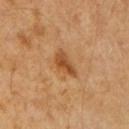| feature | finding |
|---|---|
| biopsy status | imaged on a skin check; not biopsied |
| subject | male, in their 60s |
| lesion diameter | ~3.5 mm (longest diameter) |
| acquisition | 15 mm crop, total-body photography |
| location | the right upper arm |